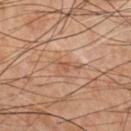Background: A roughly 15 mm field-of-view crop from a total-body skin photograph. The total-body-photography lesion software estimated a footprint of about 2.5 mm², an outline eccentricity of about 0.9 (0 = round, 1 = elongated), and two-axis asymmetry of about 0.5. And it measured an average lesion color of about L≈52 a*≈21 b*≈31 (CIELAB), roughly 8 lightness units darker than nearby skin, and a normalized lesion–skin contrast near 5.5. The software also gave a border-irregularity rating of about 5.5/10, internal color variation of about 0 on a 0–10 scale, and a peripheral color-asymmetry measure near 0. And it measured an automated nevus-likeness rating near 0 out of 100 and a detector confidence of about 95 out of 100 that the crop contains a lesion. The lesion is on the chest. Longest diameter approximately 3 mm. The tile uses cross-polarized illumination. A male subject aged 58–62.